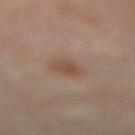Recorded during total-body skin imaging; not selected for excision or biopsy. The patient is a female roughly 50 years of age. Cropped from a total-body skin-imaging series; the visible field is about 15 mm. From the leg.